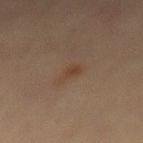<tbp_lesion>
<lighting>cross-polarized</lighting>
<patient>
  <sex>male</sex>
  <age_approx>60</age_approx>
</patient>
<site>mid back</site>
<image>
  <source>total-body photography crop</source>
  <field_of_view_mm>15</field_of_view_mm>
</image>
</tbp_lesion>